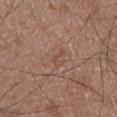{"biopsy_status": "not biopsied; imaged during a skin examination", "patient": {"sex": "male", "age_approx": 75}, "lesion_size": {"long_diameter_mm_approx": 2.5}, "lighting": "white-light", "image": {"source": "total-body photography crop", "field_of_view_mm": 15}, "site": "front of the torso", "automated_metrics": {"nevus_likeness_0_100": 0, "lesion_detection_confidence_0_100": 95}}